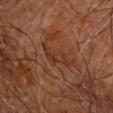Acquisition and patient details:
A roughly 15 mm field-of-view crop from a total-body skin photograph. A subject approximately 65 years of age. About 5.5 mm across. Imaged with cross-polarized lighting. The lesion-visualizer software estimated an area of roughly 6 mm², a shape eccentricity near 0.95, and a shape-asymmetry score of about 0.55 (0 = symmetric). The software also gave a mean CIELAB color near L≈34 a*≈22 b*≈29, roughly 6 lightness units darker than nearby skin, and a normalized lesion–skin contrast near 6. The lesion is located on the right upper arm.